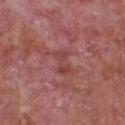Q: Is there a histopathology result?
A: imaged on a skin check; not biopsied
Q: What is the lesion's diameter?
A: ≈3 mm
Q: What lighting was used for the tile?
A: white-light
Q: How was this image acquired?
A: ~15 mm tile from a whole-body skin photo
Q: Where on the body is the lesion?
A: the front of the torso
Q: What are the patient's age and sex?
A: male, aged around 65